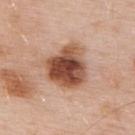{
  "biopsy_status": "not biopsied; imaged during a skin examination",
  "lesion_size": {
    "long_diameter_mm_approx": 5.0
  },
  "patient": {
    "sex": "male",
    "age_approx": 55
  },
  "image": {
    "source": "total-body photography crop",
    "field_of_view_mm": 15
  },
  "lighting": "white-light",
  "site": "upper back"
}